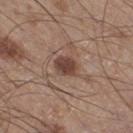Assessment:
Captured during whole-body skin photography for melanoma surveillance; the lesion was not biopsied.
Acquisition and patient details:
Located on the right thigh. The patient is a male in their 60s. A 15 mm close-up extracted from a 3D total-body photography capture.